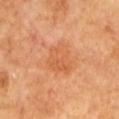Assessment: The lesion was tiled from a total-body skin photograph and was not biopsied. Clinical summary: On the left upper arm. A roughly 15 mm field-of-view crop from a total-body skin photograph. Automated tile analysis of the lesion measured a footprint of about 10 mm², an outline eccentricity of about 0.55 (0 = round, 1 = elongated), and a shape-asymmetry score of about 0.3 (0 = symmetric). It also reported a classifier nevus-likeness of about 10/100 and a lesion-detection confidence of about 100/100. The tile uses cross-polarized illumination. A male subject approximately 65 years of age. The recorded lesion diameter is about 4 mm.